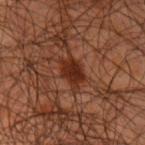{
  "biopsy_status": "not biopsied; imaged during a skin examination",
  "lighting": "cross-polarized",
  "image": {
    "source": "total-body photography crop",
    "field_of_view_mm": 15
  },
  "patient": {
    "sex": "male",
    "age_approx": 45
  },
  "site": "left upper arm"
}